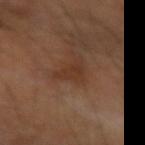Case summary:
• biopsy status — imaged on a skin check; not biopsied
• illumination — cross-polarized
• size — ~3 mm (longest diameter)
• site — the arm
• patient — male, aged around 65
• image — total-body-photography crop, ~15 mm field of view